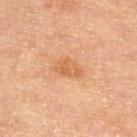Image and clinical context:
The lesion is located on the right thigh. The patient is a female in their mid-50s. A 15 mm crop from a total-body photograph taken for skin-cancer surveillance. The lesion's longest dimension is about 3.5 mm.